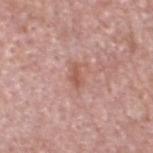workup: catalogued during a skin exam; not biopsied | anatomic site: the head or neck | image-analysis metrics: a lesion color around L≈56 a*≈24 b*≈28 in CIELAB; a border-irregularity rating of about 3.5/10, internal color variation of about 0.5 on a 0–10 scale, and peripheral color asymmetry of about 0 | lesion diameter: ≈3 mm | image: 15 mm crop, total-body photography | patient: male, aged around 70 | tile lighting: white-light.